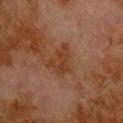  biopsy_status: not biopsied; imaged during a skin examination
  lesion_size:
    long_diameter_mm_approx: 4.0
  image:
    source: total-body photography crop
    field_of_view_mm: 15
  lighting: cross-polarized
  site: upper back
  patient:
    sex: male
    age_approx: 80